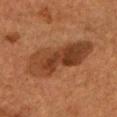<tbp_lesion>
<image>
  <source>total-body photography crop</source>
  <field_of_view_mm>15</field_of_view_mm>
</image>
<site>head or neck</site>
<patient>
  <sex>male</sex>
  <age_approx>60</age_approx>
</patient>
<lesion_size>
  <long_diameter_mm_approx>8.5</long_diameter_mm_approx>
</lesion_size>
<lighting>cross-polarized</lighting>
</tbp_lesion>